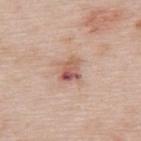Q: Was this lesion biopsied?
A: no biopsy performed (imaged during a skin exam)
Q: What is the imaging modality?
A: ~15 mm tile from a whole-body skin photo
Q: Who is the patient?
A: female, in their mid-60s
Q: Where on the body is the lesion?
A: the upper back
Q: What lighting was used for the tile?
A: white-light